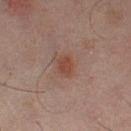{
  "biopsy_status": "not biopsied; imaged during a skin examination",
  "site": "left thigh",
  "lesion_size": {
    "long_diameter_mm_approx": 3.0
  },
  "lighting": "cross-polarized",
  "automated_metrics": {
    "area_mm2_approx": 4.5,
    "eccentricity": 0.7,
    "cielab_L": 35,
    "cielab_a": 17,
    "cielab_b": 22,
    "vs_skin_darker_L": 6.0,
    "vs_skin_contrast_norm": 6.5,
    "border_irregularity_0_10": 1.5,
    "color_variation_0_10": 1.5,
    "lesion_detection_confidence_0_100": 100
  },
  "patient": {
    "sex": "male",
    "age_approx": 45
  },
  "image": {
    "source": "total-body photography crop",
    "field_of_view_mm": 15
  }
}